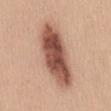{"biopsy_status": "not biopsied; imaged during a skin examination", "patient": {"sex": "female", "age_approx": 45}, "site": "mid back", "image": {"source": "total-body photography crop", "field_of_view_mm": 15}}